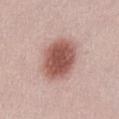The patient is a female about 30 years old. Approximately 5.5 mm at its widest. A 15 mm crop from a total-body photograph taken for skin-cancer surveillance. Imaged with white-light lighting. On the abdomen. Automated image analysis of the tile measured a footprint of about 18 mm², an outline eccentricity of about 0.7 (0 = round, 1 = elongated), and a symmetry-axis asymmetry near 0.1. The analysis additionally found a mean CIELAB color near L≈54 a*≈23 b*≈25, roughly 16 lightness units darker than nearby skin, and a normalized border contrast of about 10.5. It also reported a border-irregularity rating of about 1.5/10 and internal color variation of about 4 on a 0–10 scale.